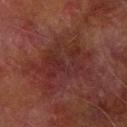Q: Was this lesion biopsied?
A: imaged on a skin check; not biopsied
Q: What kind of image is this?
A: total-body-photography crop, ~15 mm field of view
Q: What are the patient's age and sex?
A: male, aged approximately 75
Q: How was the tile lit?
A: cross-polarized
Q: Where on the body is the lesion?
A: the right forearm